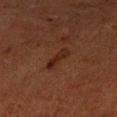Impression:
This lesion was catalogued during total-body skin photography and was not selected for biopsy.
Acquisition and patient details:
A 15 mm close-up extracted from a 3D total-body photography capture. The subject is a male approximately 60 years of age. The lesion is located on the right upper arm.